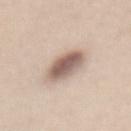Notes:
* follow-up · no biopsy performed (imaged during a skin exam)
* site · the abdomen
* acquisition · total-body-photography crop, ~15 mm field of view
* lesion size · ~4 mm (longest diameter)
* patient · female, aged 43 to 47
* TBP lesion metrics · a lesion color around L≈59 a*≈16 b*≈23 in CIELAB; a within-lesion color-variation index near 5.5/10 and a peripheral color-asymmetry measure near 1.5
* tile lighting · white-light